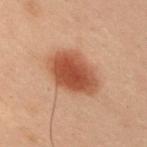Impression:
This lesion was catalogued during total-body skin photography and was not selected for biopsy.
Clinical summary:
The lesion-visualizer software estimated a lesion color around L≈41 a*≈22 b*≈29 in CIELAB and roughly 12 lightness units darker than nearby skin. A male patient about 55 years old. The lesion is located on the right upper arm. Cropped from a whole-body photographic skin survey; the tile spans about 15 mm. Captured under cross-polarized illumination.